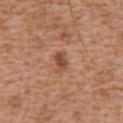A male subject, roughly 75 years of age.
The lesion is located on the chest.
Captured under white-light illumination.
Cropped from a whole-body photographic skin survey; the tile spans about 15 mm.
Measured at roughly 2.5 mm in maximum diameter.